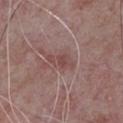biopsy status = total-body-photography surveillance lesion; no biopsy | lighting = white-light | image source = ~15 mm crop, total-body skin-cancer survey | body site = the chest | patient = male, aged 63–67 | lesion size = ~3.5 mm (longest diameter).